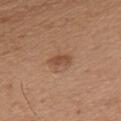| field | value |
|---|---|
| notes | catalogued during a skin exam; not biopsied |
| site | the front of the torso |
| image source | total-body-photography crop, ~15 mm field of view |
| patient | male, aged 48–52 |